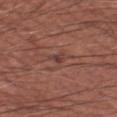biopsy status = total-body-photography surveillance lesion; no biopsy | imaging modality = ~15 mm tile from a whole-body skin photo | patient = male, aged 58–62 | diameter = ~2.5 mm (longest diameter) | location = the left thigh.